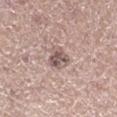Clinical impression: Recorded during total-body skin imaging; not selected for excision or biopsy. Context: The lesion is located on the leg. Longest diameter approximately 3 mm. A 15 mm close-up tile from a total-body photography series done for melanoma screening. A male patient, in their mid-60s. Imaged with white-light lighting.